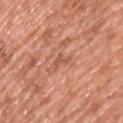notes: no biopsy performed (imaged during a skin exam)
diameter: about 3 mm
illumination: white-light
patient: male, aged 43 to 47
acquisition: total-body-photography crop, ~15 mm field of view
anatomic site: the upper back